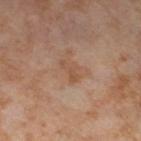biopsy status: total-body-photography surveillance lesion; no biopsy | patient: female, about 55 years old | anatomic site: the leg | tile lighting: cross-polarized illumination | automated metrics: a lesion color around L≈52 a*≈20 b*≈31 in CIELAB and a lesion-to-skin contrast of about 5.5 (normalized; higher = more distinct); border irregularity of about 5 on a 0–10 scale, internal color variation of about 0 on a 0–10 scale, and radial color variation of about 0; a nevus-likeness score of about 0/100 | image: ~15 mm crop, total-body skin-cancer survey.